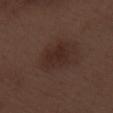Impression:
This lesion was catalogued during total-body skin photography and was not selected for biopsy.
Acquisition and patient details:
Cropped from a total-body skin-imaging series; the visible field is about 15 mm. From the arm. About 4 mm across. A male patient approximately 70 years of age. The tile uses white-light illumination.